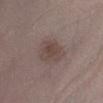The lesion was photographed on a routine skin check and not biopsied; there is no pathology result. The lesion is located on the right lower leg. A male subject aged 63–67. A lesion tile, about 15 mm wide, cut from a 3D total-body photograph. Measured at roughly 3.5 mm in maximum diameter.